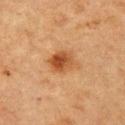| field | value |
|---|---|
| follow-up | imaged on a skin check; not biopsied |
| subject | female, aged 53 to 57 |
| lesion diameter | ≈4 mm |
| automated metrics | a footprint of about 8.5 mm² and a symmetry-axis asymmetry near 0.25; an average lesion color of about L≈43 a*≈21 b*≈34 (CIELAB), roughly 10 lightness units darker than nearby skin, and a normalized lesion–skin contrast near 8.5; a border-irregularity rating of about 2.5/10, a color-variation rating of about 6.5/10, and radial color variation of about 2.5; a nevus-likeness score of about 95/100 |
| site | the left upper arm |
| acquisition | total-body-photography crop, ~15 mm field of view |